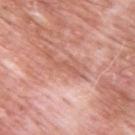<lesion>
  <biopsy_status>not biopsied; imaged during a skin examination</biopsy_status>
  <patient>
    <sex>male</sex>
    <age_approx>60</age_approx>
  </patient>
  <image>
    <source>total-body photography crop</source>
    <field_of_view_mm>15</field_of_view_mm>
  </image>
  <site>upper back</site>
  <lesion_size>
    <long_diameter_mm_approx>3.0</long_diameter_mm_approx>
  </lesion_size>
</lesion>